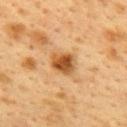<lesion>
<lighting>cross-polarized</lighting>
<image>
  <source>total-body photography crop</source>
  <field_of_view_mm>15</field_of_view_mm>
</image>
<patient>
  <sex>female</sex>
  <age_approx>40</age_approx>
</patient>
<automated_metrics>
  <area_mm2_approx>7.0</area_mm2_approx>
  <eccentricity>0.5</eccentricity>
  <shape_asymmetry>0.2</shape_asymmetry>
  <cielab_L>44</cielab_L>
  <cielab_a>20</cielab_a>
  <cielab_b>37</cielab_b>
  <vs_skin_darker_L>13.0</vs_skin_darker_L>
  <lesion_detection_confidence_0_100>100</lesion_detection_confidence_0_100>
</automated_metrics>
<site>back</site>
<lesion_size>
  <long_diameter_mm_approx>3.0</long_diameter_mm_approx>
</lesion_size>
</lesion>